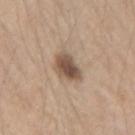Impression:
Recorded during total-body skin imaging; not selected for excision or biopsy.
Image and clinical context:
Automated image analysis of the tile measured a footprint of about 7.5 mm², a shape eccentricity near 0.55, and two-axis asymmetry of about 0.25. It also reported a lesion color around L≈51 a*≈15 b*≈27 in CIELAB and a normalized border contrast of about 9.5. Imaged with white-light lighting. Located on the left forearm. A close-up tile cropped from a whole-body skin photograph, about 15 mm across. Longest diameter approximately 3.5 mm. The subject is a female roughly 45 years of age.